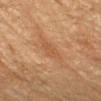Clinical impression: Part of a total-body skin-imaging series; this lesion was reviewed on a skin check and was not flagged for biopsy. Image and clinical context: Longest diameter approximately 2.5 mm. This is a cross-polarized tile. Located on the arm. A roughly 15 mm field-of-view crop from a total-body skin photograph. The patient is a female roughly 70 years of age.